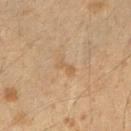<tbp_lesion>
  <image>
    <source>total-body photography crop</source>
    <field_of_view_mm>15</field_of_view_mm>
  </image>
  <patient>
    <sex>female</sex>
    <age_approx>40</age_approx>
  </patient>
  <site>right upper arm</site>
</tbp_lesion>